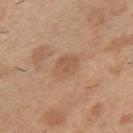Captured during whole-body skin photography for melanoma surveillance; the lesion was not biopsied.
A 15 mm close-up extracted from a 3D total-body photography capture.
Imaged with white-light lighting.
The lesion is on the left upper arm.
The total-body-photography lesion software estimated an area of roughly 7.5 mm², an outline eccentricity of about 0.65 (0 = round, 1 = elongated), and a symmetry-axis asymmetry near 0.2.
Approximately 3.5 mm at its widest.
The patient is a male aged 38–42.